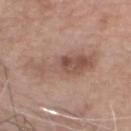Q: What is the lesion's diameter?
A: ≈8.5 mm
Q: Automated lesion metrics?
A: internal color variation of about 5.5 on a 0–10 scale and a peripheral color-asymmetry measure near 2; a nevus-likeness score of about 0/100
Q: What is the anatomic site?
A: the head or neck
Q: What lighting was used for the tile?
A: white-light illumination
Q: Patient demographics?
A: male, aged around 65
Q: What is the imaging modality?
A: 15 mm crop, total-body photography